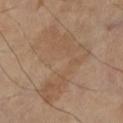| field | value |
|---|---|
| follow-up | imaged on a skin check; not biopsied |
| diameter | ≈10 mm |
| acquisition | 15 mm crop, total-body photography |
| anatomic site | the left lower leg |
| subject | female, roughly 70 years of age |